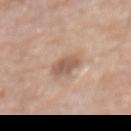Clinical impression: The lesion was photographed on a routine skin check and not biopsied; there is no pathology result. Context: The lesion is located on the abdomen. A male patient, in their mid-60s. This is a white-light tile. This image is a 15 mm lesion crop taken from a total-body photograph.